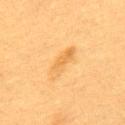Assessment: The lesion was photographed on a routine skin check and not biopsied; there is no pathology result. Background: The lesion-visualizer software estimated a border-irregularity rating of about 3.5/10, a within-lesion color-variation index near 3.5/10, and radial color variation of about 1.5. The software also gave a lesion-detection confidence of about 100/100. The lesion is on the back. A female subject, aged approximately 55. A 15 mm close-up tile from a total-body photography series done for melanoma screening. Measured at roughly 5 mm in maximum diameter. Captured under cross-polarized illumination.